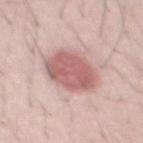body site = the abdomen; patient = male, roughly 25 years of age; image source = 15 mm crop, total-body photography.